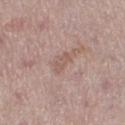<lesion>
  <biopsy_status>not biopsied; imaged during a skin examination</biopsy_status>
  <lesion_size>
    <long_diameter_mm_approx>3.0</long_diameter_mm_approx>
  </lesion_size>
  <image>
    <source>total-body photography crop</source>
    <field_of_view_mm>15</field_of_view_mm>
  </image>
  <site>leg</site>
  <lighting>white-light</lighting>
  <automated_metrics>
    <nevus_likeness_0_100>0</nevus_likeness_0_100>
  </automated_metrics>
  <patient>
    <sex>female</sex>
    <age_approx>50</age_approx>
  </patient>
</lesion>